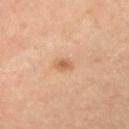<record>
  <biopsy_status>not biopsied; imaged during a skin examination</biopsy_status>
  <image>
    <source>total-body photography crop</source>
    <field_of_view_mm>15</field_of_view_mm>
  </image>
  <site>arm</site>
  <patient>
    <sex>male</sex>
    <age_approx>55</age_approx>
  </patient>
  <lighting>cross-polarized</lighting>
  <automated_metrics>
    <border_irregularity_0_10>2.5</border_irregularity_0_10>
    <color_variation_0_10>1.5</color_variation_0_10>
    <peripheral_color_asymmetry>0.5</peripheral_color_asymmetry>
    <nevus_likeness_0_100>55</nevus_likeness_0_100>
  </automated_metrics>
  <lesion_size>
    <long_diameter_mm_approx>2.0</long_diameter_mm_approx>
  </lesion_size>
</record>